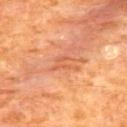Recorded during total-body skin imaging; not selected for excision or biopsy. A male subject in their mid-60s. Approximately 3.5 mm at its widest. This is a cross-polarized tile. An algorithmic analysis of the crop reported roughly 7 lightness units darker than nearby skin and a lesion-to-skin contrast of about 4.5 (normalized; higher = more distinct). Located on the upper back. Cropped from a whole-body photographic skin survey; the tile spans about 15 mm.